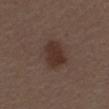Case summary:
• follow-up: catalogued during a skin exam; not biopsied
• tile lighting: white-light
• TBP lesion metrics: a lesion area of about 9.5 mm², an eccentricity of roughly 0.7, and a shape-asymmetry score of about 0.15 (0 = symmetric); a mean CIELAB color near L≈31 a*≈16 b*≈22, a lesion–skin lightness drop of about 9, and a lesion-to-skin contrast of about 8.5 (normalized; higher = more distinct); a border-irregularity rating of about 1.5/10, a color-variation rating of about 2/10, and radial color variation of about 0.5
• site: the mid back
• image: ~15 mm tile from a whole-body skin photo
• patient: male, aged 68 to 72